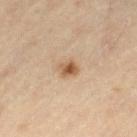Q: Is there a histopathology result?
A: imaged on a skin check; not biopsied
Q: What did automated image analysis measure?
A: a shape eccentricity near 0.6 and a shape-asymmetry score of about 0.2 (0 = symmetric); a border-irregularity index near 2/10, a color-variation rating of about 4.5/10, and radial color variation of about 1.5; a nevus-likeness score of about 95/100 and a detector confidence of about 100 out of 100 that the crop contains a lesion
Q: What kind of image is this?
A: total-body-photography crop, ~15 mm field of view
Q: Where on the body is the lesion?
A: the leg
Q: What lighting was used for the tile?
A: cross-polarized illumination
Q: Lesion size?
A: ≈2.5 mm
Q: What are the patient's age and sex?
A: male, approximately 55 years of age